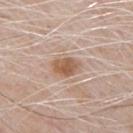Impression:
No biopsy was performed on this lesion — it was imaged during a full skin examination and was not determined to be concerning.
Clinical summary:
Cropped from a whole-body photographic skin survey; the tile spans about 15 mm. On the chest. A male patient, aged around 70.